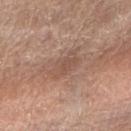| feature | finding |
|---|---|
| site | the right forearm |
| diameter | ~4 mm (longest diameter) |
| lighting | white-light illumination |
| patient | female, in their 60s |
| image | ~15 mm tile from a whole-body skin photo |
| TBP lesion metrics | a lesion area of about 6.5 mm², an outline eccentricity of about 0.85 (0 = round, 1 = elongated), and a shape-asymmetry score of about 0.25 (0 = symmetric); a lesion color around L≈52 a*≈19 b*≈26 in CIELAB and roughly 7 lightness units darker than nearby skin |